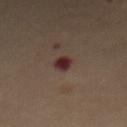follow-up — no biopsy performed (imaged during a skin exam)
illumination — cross-polarized illumination
diameter — about 2.5 mm
location — the abdomen
automated lesion analysis — a footprint of about 3.5 mm² and an outline eccentricity of about 0.65 (0 = round, 1 = elongated); a color-variation rating of about 2/10 and peripheral color asymmetry of about 0.5
acquisition — ~15 mm tile from a whole-body skin photo
patient — female, aged 63 to 67